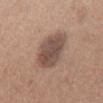| feature | finding |
|---|---|
| notes | catalogued during a skin exam; not biopsied |
| image source | ~15 mm crop, total-body skin-cancer survey |
| site | the chest |
| diameter | ≈4.5 mm |
| subject | female, aged around 55 |
| illumination | white-light illumination |
| TBP lesion metrics | an eccentricity of roughly 0.45 and a shape-asymmetry score of about 0.15 (0 = symmetric); a nevus-likeness score of about 35/100 and lesion-presence confidence of about 100/100 |